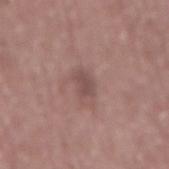Q: Was a biopsy performed?
A: no biopsy performed (imaged during a skin exam)
Q: What is the lesion's diameter?
A: about 2.5 mm
Q: Illumination type?
A: white-light
Q: What kind of image is this?
A: total-body-photography crop, ~15 mm field of view
Q: What did automated image analysis measure?
A: a lesion area of about 3 mm² and two-axis asymmetry of about 0.25
Q: Lesion location?
A: the mid back
Q: What are the patient's age and sex?
A: male, aged 58–62